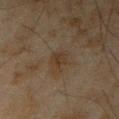From the left forearm. A male patient aged around 45. A close-up tile cropped from a whole-body skin photograph, about 15 mm across.